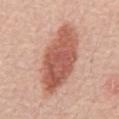The lesion is on the mid back. About 9 mm across. The patient is a male aged 68–72. This image is a 15 mm lesion crop taken from a total-body photograph.